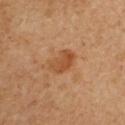{"biopsy_status": "not biopsied; imaged during a skin examination", "patient": {"sex": "male", "age_approx": 50}, "image": {"source": "total-body photography crop", "field_of_view_mm": 15}, "site": "right upper arm", "lesion_size": {"long_diameter_mm_approx": 3.0}}